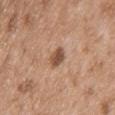This lesion was catalogued during total-body skin photography and was not selected for biopsy. The lesion is on the chest. A region of skin cropped from a whole-body photographic capture, roughly 15 mm wide. Automated tile analysis of the lesion measured a lesion area of about 4 mm², an outline eccentricity of about 0.85 (0 = round, 1 = elongated), and a symmetry-axis asymmetry near 0.3. And it measured an average lesion color of about L≈51 a*≈20 b*≈30 (CIELAB), roughly 13 lightness units darker than nearby skin, and a lesion-to-skin contrast of about 9 (normalized; higher = more distinct). The software also gave a classifier nevus-likeness of about 65/100 and a lesion-detection confidence of about 100/100. A male subject in their 50s.